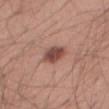On the right thigh.
A 15 mm crop from a total-body photograph taken for skin-cancer surveillance.
About 3.5 mm across.
The subject is a male aged 38–42.
Imaged with white-light lighting.
An algorithmic analysis of the crop reported border irregularity of about 2 on a 0–10 scale, a color-variation rating of about 3.5/10, and peripheral color asymmetry of about 1.